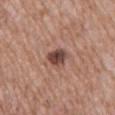Background:
A male subject aged around 60. On the abdomen. The recorded lesion diameter is about 2.5 mm. This is a white-light tile. A 15 mm crop from a total-body photograph taken for skin-cancer surveillance.